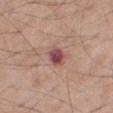Context:
The lesion is located on the right thigh. A male patient aged 68–72. Approximately 3 mm at its widest. A 15 mm close-up tile from a total-body photography series done for melanoma screening.